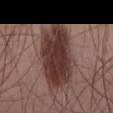Assessment: Imaged during a routine full-body skin examination; the lesion was not biopsied and no histopathology is available. Clinical summary: Located on the lower back. A male patient, in their 50s. Measured at roughly 9 mm in maximum diameter. The tile uses white-light illumination. Cropped from a whole-body photographic skin survey; the tile spans about 15 mm. Automated tile analysis of the lesion measured a lesion area of about 31 mm², an outline eccentricity of about 0.9 (0 = round, 1 = elongated), and two-axis asymmetry of about 0.2. And it measured a border-irregularity rating of about 2.5/10, a within-lesion color-variation index near 5.5/10, and peripheral color asymmetry of about 2.